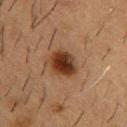| feature | finding |
|---|---|
| follow-up | total-body-photography surveillance lesion; no biopsy |
| image source | ~15 mm tile from a whole-body skin photo |
| image-analysis metrics | an average lesion color of about L≈31 a*≈19 b*≈28 (CIELAB), a lesion–skin lightness drop of about 14, and a normalized border contrast of about 12.5; a border-irregularity index near 1.5/10, internal color variation of about 5.5 on a 0–10 scale, and radial color variation of about 1.5 |
| subject | male, roughly 55 years of age |
| illumination | cross-polarized illumination |
| body site | the chest |